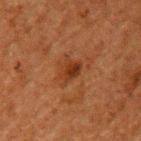Q: Is there a histopathology result?
A: no biopsy performed (imaged during a skin exam)